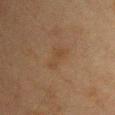Recorded during total-body skin imaging; not selected for excision or biopsy.
An algorithmic analysis of the crop reported a footprint of about 5 mm². And it measured a border-irregularity rating of about 3.5/10, a within-lesion color-variation index near 1.5/10, and peripheral color asymmetry of about 0.5. The software also gave an automated nevus-likeness rating near 0 out of 100 and a lesion-detection confidence of about 100/100.
A 15 mm crop from a total-body photograph taken for skin-cancer surveillance.
The patient is a female aged 38–42.
Located on the chest.